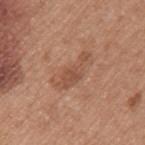Assessment: No biopsy was performed on this lesion — it was imaged during a full skin examination and was not determined to be concerning. Image and clinical context: Imaged with white-light lighting. Cropped from a whole-body photographic skin survey; the tile spans about 15 mm. Approximately 5.5 mm at its widest. The lesion-visualizer software estimated a lesion color around L≈51 a*≈22 b*≈30 in CIELAB. It also reported a classifier nevus-likeness of about 5/100. A female subject aged around 50. From the left upper arm.